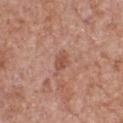biopsy status = no biopsy performed (imaged during a skin exam)
anatomic site = the chest
patient = male, aged 63–67
lesion size = about 2.5 mm
image = ~15 mm tile from a whole-body skin photo